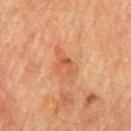Impression: The lesion was photographed on a routine skin check and not biopsied; there is no pathology result. Image and clinical context: This image is a 15 mm lesion crop taken from a total-body photograph. Imaged with cross-polarized lighting. The lesion is located on the chest. A male patient, roughly 75 years of age.